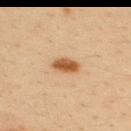Q: Was a biopsy performed?
A: imaged on a skin check; not biopsied
Q: Patient demographics?
A: male, roughly 35 years of age
Q: Where on the body is the lesion?
A: the upper back
Q: What is the imaging modality?
A: ~15 mm tile from a whole-body skin photo
Q: How was the tile lit?
A: cross-polarized
Q: How large is the lesion?
A: ≈3 mm